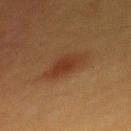Part of a total-body skin-imaging series; this lesion was reviewed on a skin check and was not flagged for biopsy. A 15 mm crop from a total-body photograph taken for skin-cancer surveillance. On the mid back. Imaged with cross-polarized lighting. The recorded lesion diameter is about 4.5 mm. A female subject, aged approximately 40.